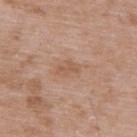Q: Was this lesion biopsied?
A: imaged on a skin check; not biopsied
Q: How was this image acquired?
A: 15 mm crop, total-body photography
Q: What is the anatomic site?
A: the back
Q: Illumination type?
A: white-light illumination
Q: How large is the lesion?
A: ≈3 mm
Q: What are the patient's age and sex?
A: male, roughly 50 years of age